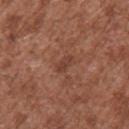image source — ~15 mm tile from a whole-body skin photo; location — the back; diameter — ~2.5 mm (longest diameter); lighting — white-light; patient — male, about 45 years old.